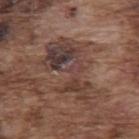| key | value |
|---|---|
| lesion size | ≈8.5 mm |
| patient | male, approximately 75 years of age |
| illumination | white-light |
| anatomic site | the upper back |
| acquisition | 15 mm crop, total-body photography |